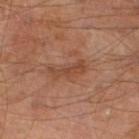workup: catalogued during a skin exam; not biopsied
image source: ~15 mm crop, total-body skin-cancer survey
illumination: cross-polarized illumination
site: the left lower leg
patient: male, in their mid-60s
size: ≈4 mm